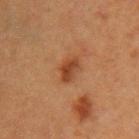- follow-up — catalogued during a skin exam; not biopsied
- image source — 15 mm crop, total-body photography
- anatomic site — the left upper arm
- patient — female, aged around 40